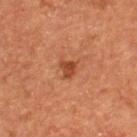This lesion was catalogued during total-body skin photography and was not selected for biopsy.
An algorithmic analysis of the crop reported an average lesion color of about L≈41 a*≈26 b*≈34 (CIELAB) and a lesion–skin lightness drop of about 10. The analysis additionally found a border-irregularity rating of about 3/10 and a peripheral color-asymmetry measure near 1.
This image is a 15 mm lesion crop taken from a total-body photograph.
Longest diameter approximately 2 mm.
The tile uses cross-polarized illumination.
On the back.
A male patient in their mid-50s.